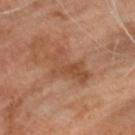biopsy status=catalogued during a skin exam; not biopsied
anatomic site=the head or neck
automated lesion analysis=an average lesion color of about L≈47 a*≈22 b*≈32 (CIELAB) and roughly 7 lightness units darker than nearby skin; a border-irregularity index near 7/10, a color-variation rating of about 3.5/10, and radial color variation of about 1.5
image source=15 mm crop, total-body photography
illumination=cross-polarized illumination
patient=male, about 70 years old
lesion diameter=~5 mm (longest diameter)